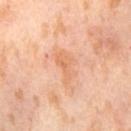An algorithmic analysis of the crop reported a symmetry-axis asymmetry near 0.45. The software also gave a mean CIELAB color near L≈70 a*≈25 b*≈38 and a lesion-to-skin contrast of about 5.5 (normalized; higher = more distinct). The analysis additionally found a nevus-likeness score of about 0/100 and a detector confidence of about 100 out of 100 that the crop contains a lesion. Approximately 4 mm at its widest. A lesion tile, about 15 mm wide, cut from a 3D total-body photograph. The patient is a female aged around 55. The lesion is located on the right thigh.